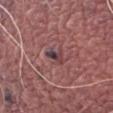{"biopsy_status": "not biopsied; imaged during a skin examination", "image": {"source": "total-body photography crop", "field_of_view_mm": 15}, "lesion_size": {"long_diameter_mm_approx": 3.0}, "site": "left thigh", "patient": {"sex": "male", "age_approx": 80}, "automated_metrics": {"vs_skin_darker_L": 10.0, "vs_skin_contrast_norm": 9.0, "color_variation_0_10": 7.5}, "lighting": "white-light"}